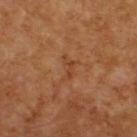• follow-up: catalogued during a skin exam; not biopsied
• acquisition: total-body-photography crop, ~15 mm field of view
• lighting: cross-polarized illumination
• site: the upper back
• subject: male, aged 58 to 62